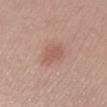{
  "biopsy_status": "not biopsied; imaged during a skin examination",
  "site": "right lower leg",
  "automated_metrics": {
    "eccentricity": 0.65,
    "shape_asymmetry": 0.15,
    "cielab_L": 56,
    "cielab_a": 22,
    "cielab_b": 25,
    "vs_skin_darker_L": 7.0,
    "vs_skin_contrast_norm": 5.0,
    "color_variation_0_10": 1.5,
    "peripheral_color_asymmetry": 0.5
  },
  "lesion_size": {
    "long_diameter_mm_approx": 3.0
  },
  "lighting": "white-light",
  "patient": {
    "sex": "female",
    "age_approx": 25
  },
  "image": {
    "source": "total-body photography crop",
    "field_of_view_mm": 15
  }
}